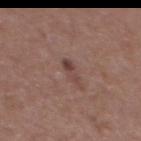Notes:
– workup: total-body-photography surveillance lesion; no biopsy
– lighting: white-light
– image: total-body-photography crop, ~15 mm field of view
– subject: male, aged approximately 55
– location: the chest
– TBP lesion metrics: an average lesion color of about L≈43 a*≈19 b*≈22 (CIELAB) and a normalized border contrast of about 6.5